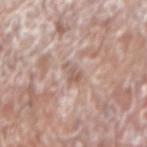Assessment: Captured during whole-body skin photography for melanoma surveillance; the lesion was not biopsied. Context: Imaged with white-light lighting. About 3 mm across. The patient is a male roughly 75 years of age. A lesion tile, about 15 mm wide, cut from a 3D total-body photograph. Located on the left forearm.